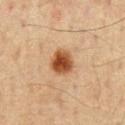workup: catalogued during a skin exam; not biopsied | image: ~15 mm tile from a whole-body skin photo | lighting: cross-polarized illumination | patient: male, roughly 60 years of age.